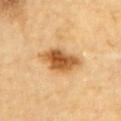Impression: This lesion was catalogued during total-body skin photography and was not selected for biopsy. Context: Captured under cross-polarized illumination. Approximately 6 mm at its widest. A male patient in their mid- to late 80s or older. A 15 mm close-up tile from a total-body photography series done for melanoma screening. Automated tile analysis of the lesion measured internal color variation of about 6 on a 0–10 scale and peripheral color asymmetry of about 2. And it measured an automated nevus-likeness rating near 95 out of 100 and lesion-presence confidence of about 100/100. The lesion is on the upper back.